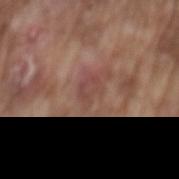workup — catalogued during a skin exam; not biopsied
image source — total-body-photography crop, ~15 mm field of view
subject — male, in their mid-70s
site — the back